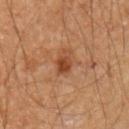This lesion was catalogued during total-body skin photography and was not selected for biopsy.
Located on the arm.
A male subject, about 60 years old.
A roughly 15 mm field-of-view crop from a total-body skin photograph.